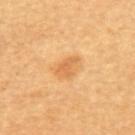Background:
The tile uses cross-polarized illumination. Longest diameter approximately 3 mm. A subject aged around 60. On the back. A 15 mm crop from a total-body photograph taken for skin-cancer surveillance.